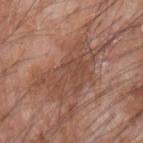The lesion was photographed on a routine skin check and not biopsied; there is no pathology result. The patient is a male aged around 60. A 15 mm crop from a total-body photograph taken for skin-cancer surveillance. From the left forearm. Longest diameter approximately 9.5 mm. The lesion-visualizer software estimated a border-irregularity rating of about 6/10, a within-lesion color-variation index near 3.5/10, and peripheral color asymmetry of about 1. It also reported an automated nevus-likeness rating near 0 out of 100 and a lesion-detection confidence of about 80/100.